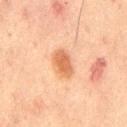biopsy_status: not biopsied; imaged during a skin examination
image:
  source: total-body photography crop
  field_of_view_mm: 15
lighting: cross-polarized
site: mid back
patient:
  sex: male
  age_approx: 65